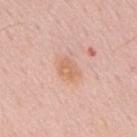This lesion was catalogued during total-body skin photography and was not selected for biopsy. Automated image analysis of the tile measured a shape eccentricity near 0.65 and a shape-asymmetry score of about 0.25 (0 = symmetric). The software also gave border irregularity of about 2.5 on a 0–10 scale and radial color variation of about 1. The software also gave an automated nevus-likeness rating near 0 out of 100. Captured under white-light illumination. A male patient about 55 years old. From the mid back. Measured at roughly 3 mm in maximum diameter. A roughly 15 mm field-of-view crop from a total-body skin photograph.